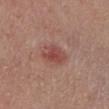{"biopsy_status": "not biopsied; imaged during a skin examination", "image": {"source": "total-body photography crop", "field_of_view_mm": 15}, "site": "left lower leg", "lesion_size": {"long_diameter_mm_approx": 3.5}, "lighting": "white-light", "patient": {"sex": "female", "age_approx": 65}}